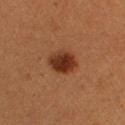Impression: This lesion was catalogued during total-body skin photography and was not selected for biopsy. Image and clinical context: The lesion is located on the right thigh. An algorithmic analysis of the crop reported a border-irregularity index near 1/10, a color-variation rating of about 4/10, and peripheral color asymmetry of about 1. The tile uses cross-polarized illumination. A region of skin cropped from a whole-body photographic capture, roughly 15 mm wide. Approximately 3.5 mm at its widest. A female subject, in their 40s.